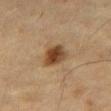- workup — imaged on a skin check; not biopsied
- anatomic site — the right thigh
- image — total-body-photography crop, ~15 mm field of view
- patient — female, about 55 years old